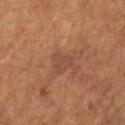Part of a total-body skin-imaging series; this lesion was reviewed on a skin check and was not flagged for biopsy.
A female subject roughly 80 years of age.
The total-body-photography lesion software estimated a footprint of about 5.5 mm², an outline eccentricity of about 0.65 (0 = round, 1 = elongated), and a shape-asymmetry score of about 0.45 (0 = symmetric). The analysis additionally found a lesion color around L≈33 a*≈17 b*≈22 in CIELAB and a lesion-to-skin contrast of about 5 (normalized; higher = more distinct).
A region of skin cropped from a whole-body photographic capture, roughly 15 mm wide.
On the right lower leg.
Longest diameter approximately 3 mm.
Imaged with cross-polarized lighting.